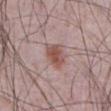follow-up: catalogued during a skin exam; not biopsied | image source: 15 mm crop, total-body photography | image-analysis metrics: border irregularity of about 2 on a 0–10 scale, internal color variation of about 3.5 on a 0–10 scale, and peripheral color asymmetry of about 1 | patient: male, aged around 65 | tile lighting: white-light illumination | body site: the abdomen.